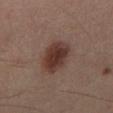Imaged during a routine full-body skin examination; the lesion was not biopsied and no histopathology is available. A male subject, aged approximately 40. The lesion is located on the right lower leg. A close-up tile cropped from a whole-body skin photograph, about 15 mm across. The lesion-visualizer software estimated an area of roughly 13 mm², an eccentricity of roughly 0.6, and two-axis asymmetry of about 0.15. And it measured a border-irregularity index near 1.5/10 and a within-lesion color-variation index near 3.5/10. The tile uses cross-polarized illumination. Measured at roughly 4.5 mm in maximum diameter.